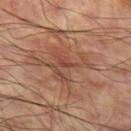The lesion was photographed on a routine skin check and not biopsied; there is no pathology result.